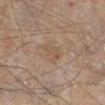Imaged during a routine full-body skin examination; the lesion was not biopsied and no histopathology is available.